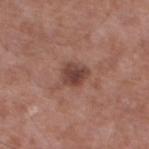imaging modality = 15 mm crop, total-body photography; tile lighting = white-light illumination; lesion size = ~3 mm (longest diameter); patient = male, aged 53 to 57; site = the left lower leg.